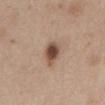Notes:
- subject: female, aged 38–42
- diameter: ≈3.5 mm
- acquisition: ~15 mm crop, total-body skin-cancer survey
- location: the front of the torso